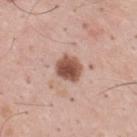workup = imaged on a skin check; not biopsied
imaging modality = ~15 mm tile from a whole-body skin photo
anatomic site = the mid back
subject = male, in their mid-30s
illumination = white-light